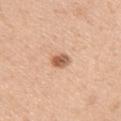notes: imaged on a skin check; not biopsied
automated lesion analysis: a lesion color around L≈60 a*≈22 b*≈34 in CIELAB, roughly 14 lightness units darker than nearby skin, and a lesion-to-skin contrast of about 8.5 (normalized; higher = more distinct); a color-variation rating of about 5.5/10 and peripheral color asymmetry of about 2; a nevus-likeness score of about 95/100 and a detector confidence of about 100 out of 100 that the crop contains a lesion
site: the arm
image source: ~15 mm crop, total-body skin-cancer survey
patient: female, about 40 years old
diameter: ~2.5 mm (longest diameter)